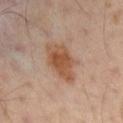notes: catalogued during a skin exam; not biopsied | subject: male, aged around 50 | anatomic site: the left arm | imaging modality: ~15 mm crop, total-body skin-cancer survey.